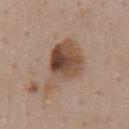Q: Was a biopsy performed?
A: imaged on a skin check; not biopsied
Q: How was this image acquired?
A: total-body-photography crop, ~15 mm field of view
Q: What lighting was used for the tile?
A: white-light illumination
Q: Where on the body is the lesion?
A: the chest
Q: What are the patient's age and sex?
A: male, about 60 years old
Q: How large is the lesion?
A: about 7 mm
Q: Automated lesion metrics?
A: an automated nevus-likeness rating near 80 out of 100 and lesion-presence confidence of about 100/100